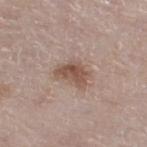This lesion was catalogued during total-body skin photography and was not selected for biopsy.
A male patient roughly 80 years of age.
Imaged with white-light lighting.
Automated image analysis of the tile measured a lesion color around L≈52 a*≈18 b*≈25 in CIELAB and roughly 11 lightness units darker than nearby skin. It also reported border irregularity of about 3.5 on a 0–10 scale and a peripheral color-asymmetry measure near 1.5.
Cropped from a total-body skin-imaging series; the visible field is about 15 mm.
Located on the left lower leg.
Approximately 3.5 mm at its widest.